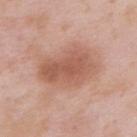This lesion was catalogued during total-body skin photography and was not selected for biopsy. A male patient roughly 55 years of age. Measured at roughly 6.5 mm in maximum diameter. A 15 mm crop from a total-body photograph taken for skin-cancer surveillance. Located on the chest. Captured under white-light illumination.